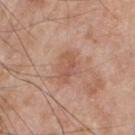Notes:
– follow-up · catalogued during a skin exam; not biopsied
– imaging modality · total-body-photography crop, ~15 mm field of view
– body site · the chest
– lighting · white-light
– patient · male, about 50 years old
– automated metrics · an average lesion color of about L≈56 a*≈22 b*≈29 (CIELAB); a classifier nevus-likeness of about 0/100 and a lesion-detection confidence of about 100/100
– size · ~4 mm (longest diameter)